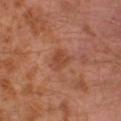Impression:
Part of a total-body skin-imaging series; this lesion was reviewed on a skin check and was not flagged for biopsy.
Acquisition and patient details:
The lesion is on the left leg. A male patient aged 28 to 32. Captured under cross-polarized illumination. The total-body-photography lesion software estimated a lesion area of about 5 mm², an outline eccentricity of about 0.65 (0 = round, 1 = elongated), and two-axis asymmetry of about 0.3. And it measured internal color variation of about 2 on a 0–10 scale and radial color variation of about 1. The analysis additionally found a classifier nevus-likeness of about 0/100 and a lesion-detection confidence of about 100/100. The recorded lesion diameter is about 3 mm. This image is a 15 mm lesion crop taken from a total-body photograph.